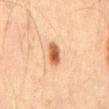Measured at roughly 3 mm in maximum diameter.
A male subject about 70 years old.
On the abdomen.
A roughly 15 mm field-of-view crop from a total-body skin photograph.
An algorithmic analysis of the crop reported roughly 14 lightness units darker than nearby skin and a lesion-to-skin contrast of about 10 (normalized; higher = more distinct). It also reported a border-irregularity index near 2.5/10. The analysis additionally found a nevus-likeness score of about 100/100 and a detector confidence of about 100 out of 100 that the crop contains a lesion.
This is a cross-polarized tile.